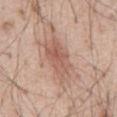notes=total-body-photography surveillance lesion; no biopsy | lesion size=~5.5 mm (longest diameter) | location=the abdomen | illumination=white-light | imaging modality=total-body-photography crop, ~15 mm field of view | patient=male, aged 53–57.